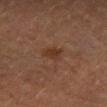Part of a total-body skin-imaging series; this lesion was reviewed on a skin check and was not flagged for biopsy. The tile uses cross-polarized illumination. The patient is a female roughly 60 years of age. The lesion is located on the left forearm. Approximately 2.5 mm at its widest. A close-up tile cropped from a whole-body skin photograph, about 15 mm across.